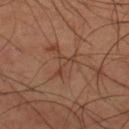biopsy status=no biopsy performed (imaged during a skin exam); site=the upper back; subject=male, aged approximately 45; size=≈3 mm; illumination=cross-polarized; acquisition=~15 mm crop, total-body skin-cancer survey.